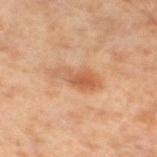Q: Was a biopsy performed?
A: no biopsy performed (imaged during a skin exam)
Q: Illumination type?
A: cross-polarized illumination
Q: What is the anatomic site?
A: the left lower leg
Q: How was this image acquired?
A: ~15 mm tile from a whole-body skin photo
Q: What is the lesion's diameter?
A: about 5 mm
Q: Who is the patient?
A: male, aged 58–62